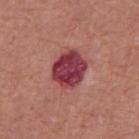{
  "lesion_size": {
    "long_diameter_mm_approx": 4.0
  },
  "image": {
    "source": "total-body photography crop",
    "field_of_view_mm": 15
  },
  "site": "chest",
  "patient": {
    "sex": "male",
    "age_approx": 65
  }
}